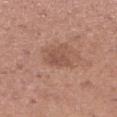Clinical impression: Recorded during total-body skin imaging; not selected for excision or biopsy. Image and clinical context: Approximately 3 mm at its widest. The patient is a female aged 28–32. A 15 mm close-up tile from a total-body photography series done for melanoma screening. The lesion is located on the right lower leg. The lesion-visualizer software estimated a footprint of about 4.5 mm², an outline eccentricity of about 0.6 (0 = round, 1 = elongated), and a shape-asymmetry score of about 0.3 (0 = symmetric). The analysis additionally found an automated nevus-likeness rating near 0 out of 100 and a detector confidence of about 100 out of 100 that the crop contains a lesion.